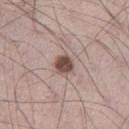This lesion was catalogued during total-body skin photography and was not selected for biopsy. Captured under white-light illumination. On the left thigh. A male patient aged 68–72. Longest diameter approximately 2.5 mm. A 15 mm close-up tile from a total-body photography series done for melanoma screening.